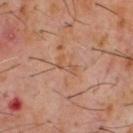Part of a total-body skin-imaging series; this lesion was reviewed on a skin check and was not flagged for biopsy. This is a cross-polarized tile. A male subject, about 60 years old. A 15 mm crop from a total-body photograph taken for skin-cancer surveillance. The total-body-photography lesion software estimated a lesion area of about 2.5 mm², an outline eccentricity of about 0.9 (0 = round, 1 = elongated), and two-axis asymmetry of about 0.55. The software also gave a mean CIELAB color near L≈49 a*≈21 b*≈31 and a lesion–skin lightness drop of about 6. The analysis additionally found border irregularity of about 7 on a 0–10 scale, internal color variation of about 0 on a 0–10 scale, and a peripheral color-asymmetry measure near 0. And it measured a classifier nevus-likeness of about 0/100 and a lesion-detection confidence of about 95/100. The lesion is located on the chest. Approximately 3 mm at its widest.